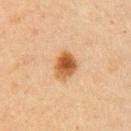workup — imaged on a skin check; not biopsied | image — 15 mm crop, total-body photography | illumination — cross-polarized illumination | lesion diameter — ≈3.5 mm | subject — female, aged around 45 | body site — the arm.